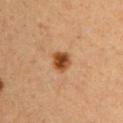Clinical impression:
The lesion was tiled from a total-body skin photograph and was not biopsied.
Image and clinical context:
Longest diameter approximately 2.5 mm. Captured under cross-polarized illumination. A 15 mm close-up tile from a total-body photography series done for melanoma screening. The subject is a female approximately 40 years of age. Automated image analysis of the tile measured a nevus-likeness score of about 100/100 and a lesion-detection confidence of about 100/100. The lesion is on the chest.